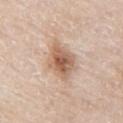Recorded during total-body skin imaging; not selected for excision or biopsy. A male patient aged approximately 80. Captured under white-light illumination. Longest diameter approximately 4 mm. A 15 mm close-up tile from a total-body photography series done for melanoma screening. On the left thigh.